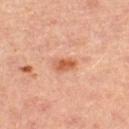Assessment:
No biopsy was performed on this lesion — it was imaged during a full skin examination and was not determined to be concerning.
Background:
The patient is a female roughly 45 years of age. Located on the leg. A lesion tile, about 15 mm wide, cut from a 3D total-body photograph.